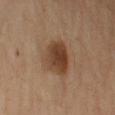notes: no biopsy performed (imaged during a skin exam)
tile lighting: cross-polarized illumination
automated metrics: a lesion color around L≈36 a*≈17 b*≈28 in CIELAB, a lesion–skin lightness drop of about 11, and a normalized border contrast of about 9.5; an automated nevus-likeness rating near 90 out of 100 and a lesion-detection confidence of about 100/100
size: ≈4.5 mm
acquisition: ~15 mm crop, total-body skin-cancer survey
site: the left upper arm
patient: male, aged around 55